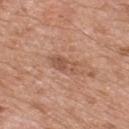biopsy_status: not biopsied; imaged during a skin examination
automated_metrics:
  eccentricity: 0.85
  shape_asymmetry: 0.35
  cielab_L: 53
  cielab_a: 22
  cielab_b: 29
  vs_skin_darker_L: 9.0
  vs_skin_contrast_norm: 6.0
  border_irregularity_0_10: 4.0
lighting: white-light
image:
  source: total-body photography crop
  field_of_view_mm: 15
site: mid back
patient:
  sex: male
  age_approx: 50
lesion_size:
  long_diameter_mm_approx: 3.5